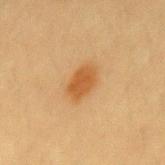patient=female, about 20 years old; image=~15 mm tile from a whole-body skin photo; body site=the mid back.